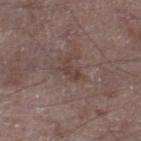Part of a total-body skin-imaging series; this lesion was reviewed on a skin check and was not flagged for biopsy.